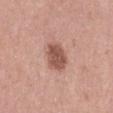The lesion is on the left thigh.
Cropped from a whole-body photographic skin survey; the tile spans about 15 mm.
The recorded lesion diameter is about 3 mm.
A female patient aged approximately 40.
The tile uses white-light illumination.